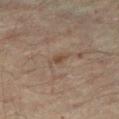| field | value |
|---|---|
| biopsy status | no biopsy performed (imaged during a skin exam) |
| size | ~2.5 mm (longest diameter) |
| illumination | cross-polarized illumination |
| patient | male, aged 43 to 47 |
| image source | ~15 mm tile from a whole-body skin photo |
| body site | the right thigh |